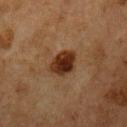| feature | finding |
|---|---|
| notes | no biopsy performed (imaged during a skin exam) |
| image | 15 mm crop, total-body photography |
| subject | male, roughly 75 years of age |
| diameter | ≈4 mm |
| site | the chest |
| lighting | cross-polarized illumination |